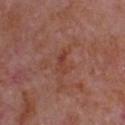No biopsy was performed on this lesion — it was imaged during a full skin examination and was not determined to be concerning.
A male patient, aged around 65.
Captured under white-light illumination.
The total-body-photography lesion software estimated about 7 CIELAB-L* units darker than the surrounding skin and a normalized border contrast of about 6. It also reported an automated nevus-likeness rating near 0 out of 100 and lesion-presence confidence of about 100/100.
About 3 mm across.
A region of skin cropped from a whole-body photographic capture, roughly 15 mm wide.
The lesion is on the chest.